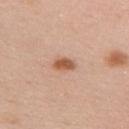Captured during whole-body skin photography for melanoma surveillance; the lesion was not biopsied. The total-body-photography lesion software estimated roughly 12 lightness units darker than nearby skin and a normalized lesion–skin contrast near 8.5. Captured under white-light illumination. A female subject approximately 35 years of age. Located on the right upper arm. Cropped from a whole-body photographic skin survey; the tile spans about 15 mm. The lesion's longest dimension is about 2.5 mm.